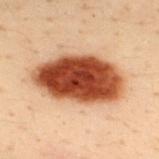<record>
<biopsy_status>not biopsied; imaged during a skin examination</biopsy_status>
<image>
  <source>total-body photography crop</source>
  <field_of_view_mm>15</field_of_view_mm>
</image>
<site>upper back</site>
<patient>
  <sex>male</sex>
  <age_approx>30</age_approx>
</patient>
</record>